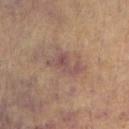This lesion was catalogued during total-body skin photography and was not selected for biopsy. A region of skin cropped from a whole-body photographic capture, roughly 15 mm wide. About 4.5 mm across. A male subject approximately 60 years of age. This is a white-light tile. The lesion is on the right forearm.